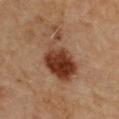Impression: The lesion was tiled from a total-body skin photograph and was not biopsied. Acquisition and patient details: The lesion is on the upper back. Captured under cross-polarized illumination. A male subject, aged 83 or older. A 15 mm close-up extracted from a 3D total-body photography capture. Measured at roughly 6.5 mm in maximum diameter.